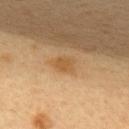TBP lesion metrics: a classifier nevus-likeness of about 5/100 and lesion-presence confidence of about 100/100
patient: female, aged approximately 45
lesion size: about 2.5 mm
body site: the upper back
acquisition: ~15 mm tile from a whole-body skin photo
tile lighting: cross-polarized illumination